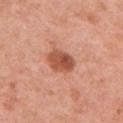follow-up=imaged on a skin check; not biopsied
location=the left upper arm
tile lighting=white-light
acquisition=15 mm crop, total-body photography
subject=female, aged 48 to 52
automated lesion analysis=a footprint of about 8 mm² and a shape eccentricity near 0.65; a color-variation rating of about 5/10 and a peripheral color-asymmetry measure near 2; a detector confidence of about 100 out of 100 that the crop contains a lesion